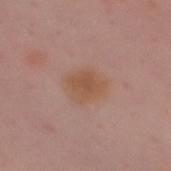Findings:
• lighting — white-light illumination
• automated metrics — a mean CIELAB color near L≈53 a*≈20 b*≈29, about 6 CIELAB-L* units darker than the surrounding skin, and a normalized lesion–skin contrast near 7; lesion-presence confidence of about 100/100
• anatomic site — the chest
• acquisition — ~15 mm crop, total-body skin-cancer survey
• subject — female, about 50 years old